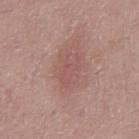Q: Is there a histopathology result?
A: catalogued during a skin exam; not biopsied